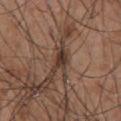Clinical impression: Recorded during total-body skin imaging; not selected for excision or biopsy. Context: A lesion tile, about 15 mm wide, cut from a 3D total-body photograph. The total-body-photography lesion software estimated a lesion-to-skin contrast of about 10.5 (normalized; higher = more distinct). Approximately 3 mm at its widest. The tile uses white-light illumination. A male subject aged approximately 55. The lesion is on the chest.